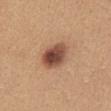workup — imaged on a skin check; not biopsied
acquisition — ~15 mm tile from a whole-body skin photo
lesion diameter — about 4.5 mm
tile lighting — white-light illumination
site — the abdomen
subject — female, aged approximately 25